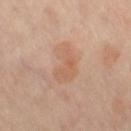workup — imaged on a skin check; not biopsied | location — the right thigh | patient — female, roughly 50 years of age | acquisition — total-body-photography crop, ~15 mm field of view.